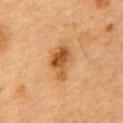Clinical summary:
Approximately 4 mm at its widest. Cropped from a whole-body photographic skin survey; the tile spans about 15 mm. The tile uses cross-polarized illumination. The subject is a male aged around 85 or older. Automated image analysis of the tile measured a mean CIELAB color near L≈46 a*≈22 b*≈38, roughly 12 lightness units darker than nearby skin, and a normalized lesion–skin contrast near 9. Located on the chest.